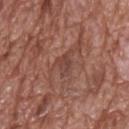  biopsy_status: not biopsied; imaged during a skin examination
  patient:
    sex: male
    age_approx: 70
  lighting: white-light
  automated_metrics:
    cielab_L: 43
    cielab_a: 21
    cielab_b: 25
    vs_skin_darker_L: 7.0
    border_irregularity_0_10: 3.5
    color_variation_0_10: 3.0
    peripheral_color_asymmetry: 1.0
  lesion_size:
    long_diameter_mm_approx: 3.0
  image:
    source: total-body photography crop
    field_of_view_mm: 15
  site: upper back